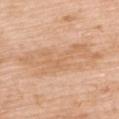Recorded during total-body skin imaging; not selected for excision or biopsy.